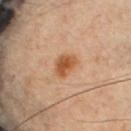Imaged during a routine full-body skin examination; the lesion was not biopsied and no histopathology is available.
The patient is a male about 40 years old.
About 3 mm across.
A 15 mm close-up extracted from a 3D total-body photography capture.
From the chest.